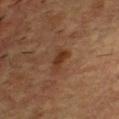This lesion was catalogued during total-body skin photography and was not selected for biopsy. Measured at roughly 3.5 mm in maximum diameter. On the upper back. A male patient about 55 years old. A 15 mm close-up extracted from a 3D total-body photography capture. Imaged with cross-polarized lighting.